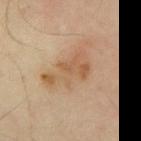Notes:
* biopsy status · imaged on a skin check; not biopsied
* diameter · ≈5.5 mm
* site · the back
* image · ~15 mm crop, total-body skin-cancer survey
* patient · male, in their mid- to late 60s
* image-analysis metrics · a mean CIELAB color near L≈53 a*≈17 b*≈33, about 8 CIELAB-L* units darker than the surrounding skin, and a lesion-to-skin contrast of about 6.5 (normalized; higher = more distinct); a border-irregularity rating of about 7/10, internal color variation of about 3 on a 0–10 scale, and peripheral color asymmetry of about 1; an automated nevus-likeness rating near 10 out of 100 and lesion-presence confidence of about 100/100
* lighting · cross-polarized